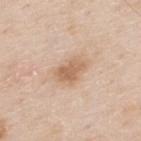Assessment: The lesion was photographed on a routine skin check and not biopsied; there is no pathology result. Image and clinical context: Located on the back. This image is a 15 mm lesion crop taken from a total-body photograph. A male patient roughly 80 years of age. About 4 mm across.